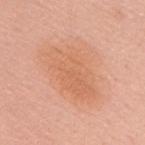Q: Is there a histopathology result?
A: catalogued during a skin exam; not biopsied